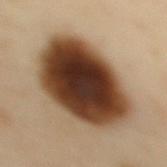workup: total-body-photography surveillance lesion; no biopsy.